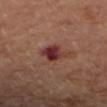workup: imaged on a skin check; not biopsied | subject: female, aged approximately 65 | body site: the right forearm | tile lighting: cross-polarized | image source: total-body-photography crop, ~15 mm field of view.